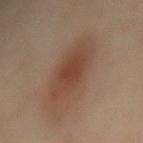Captured during whole-body skin photography for melanoma surveillance; the lesion was not biopsied. Approximately 8 mm at its widest. This is a cross-polarized tile. The patient is a female roughly 55 years of age. The total-body-photography lesion software estimated an area of roughly 18 mm². The software also gave a lesion color around L≈44 a*≈19 b*≈28 in CIELAB and a normalized border contrast of about 8. The software also gave a border-irregularity index near 3/10, a color-variation rating of about 3/10, and radial color variation of about 0.5. The analysis additionally found a classifier nevus-likeness of about 100/100 and a lesion-detection confidence of about 100/100. On the mid back. This image is a 15 mm lesion crop taken from a total-body photograph.